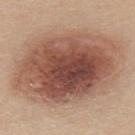Q: Was a biopsy performed?
A: catalogued during a skin exam; not biopsied
Q: What did automated image analysis measure?
A: a border-irregularity rating of about 1.5/10 and a peripheral color-asymmetry measure near 2.5
Q: How was the tile lit?
A: white-light
Q: Patient demographics?
A: female, approximately 40 years of age
Q: Where on the body is the lesion?
A: the upper back
Q: How was this image acquired?
A: total-body-photography crop, ~15 mm field of view